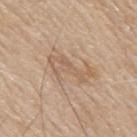  biopsy_status: not biopsied; imaged during a skin examination